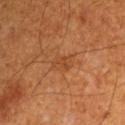Notes:
- follow-up: catalogued during a skin exam; not biopsied
- patient: male, in their 60s
- body site: the left upper arm
- lesion diameter: ~2.5 mm (longest diameter)
- tile lighting: cross-polarized illumination
- automated metrics: a footprint of about 4 mm² and an outline eccentricity of about 0.7 (0 = round, 1 = elongated); a border-irregularity rating of about 2.5/10 and peripheral color asymmetry of about 0.5
- imaging modality: ~15 mm crop, total-body skin-cancer survey